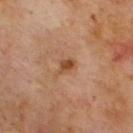This lesion was catalogued during total-body skin photography and was not selected for biopsy.
Cropped from a whole-body photographic skin survey; the tile spans about 15 mm.
From the upper back.
A male patient aged approximately 70.